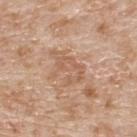biopsy status: total-body-photography surveillance lesion; no biopsy
image-analysis metrics: an area of roughly 8.5 mm², a shape eccentricity near 0.85, and a shape-asymmetry score of about 0.4 (0 = symmetric); an average lesion color of about L≈59 a*≈20 b*≈32 (CIELAB) and a normalized lesion–skin contrast near 5; a border-irregularity index near 5.5/10
location: the upper back
imaging modality: ~15 mm crop, total-body skin-cancer survey
diameter: about 4.5 mm
patient: male, aged 78 to 82